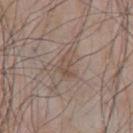| feature | finding |
|---|---|
| workup | catalogued during a skin exam; not biopsied |
| diameter | ≈3.5 mm |
| site | the chest |
| imaging modality | total-body-photography crop, ~15 mm field of view |
| automated lesion analysis | an area of roughly 6 mm², an eccentricity of roughly 0.8, and a symmetry-axis asymmetry near 0.4; a mean CIELAB color near L≈50 a*≈14 b*≈24 and about 7 CIELAB-L* units darker than the surrounding skin; a border-irregularity index near 4/10 and internal color variation of about 3.5 on a 0–10 scale; an automated nevus-likeness rating near 0 out of 100 and lesion-presence confidence of about 95/100 |
| subject | male, in their mid-60s |